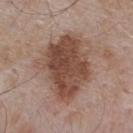Clinical impression: The lesion was photographed on a routine skin check and not biopsied; there is no pathology result. Clinical summary: Automated tile analysis of the lesion measured border irregularity of about 3 on a 0–10 scale, a color-variation rating of about 6/10, and peripheral color asymmetry of about 2. It also reported a nevus-likeness score of about 20/100. Imaged with white-light lighting. A close-up tile cropped from a whole-body skin photograph, about 15 mm across. The patient is a male aged 53 to 57. On the upper back.